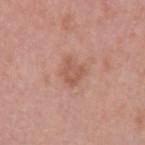Recorded during total-body skin imaging; not selected for excision or biopsy. The lesion is on the left upper arm. Cropped from a total-body skin-imaging series; the visible field is about 15 mm. Captured under white-light illumination. A female subject, aged 43–47. The recorded lesion diameter is about 3 mm. Automated image analysis of the tile measured a lesion–skin lightness drop of about 8 and a normalized lesion–skin contrast near 5.5. It also reported a within-lesion color-variation index near 2.5/10 and a peripheral color-asymmetry measure near 1. It also reported a nevus-likeness score of about 5/100 and lesion-presence confidence of about 100/100.